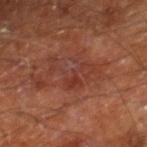| feature | finding |
|---|---|
| workup | no biopsy performed (imaged during a skin exam) |
| acquisition | ~15 mm tile from a whole-body skin photo |
| automated metrics | a lesion area of about 9.5 mm² and a shape eccentricity near 0.8; a mean CIELAB color near L≈36 a*≈25 b*≈27 and a lesion–skin lightness drop of about 6; a border-irregularity index near 10/10, internal color variation of about 3.5 on a 0–10 scale, and peripheral color asymmetry of about 0.5; a classifier nevus-likeness of about 0/100 and lesion-presence confidence of about 100/100 |
| anatomic site | the leg |
| diameter | about 5.5 mm |
| tile lighting | cross-polarized |
| subject | male, aged 58 to 62 |